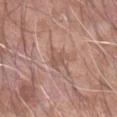Clinical impression: Recorded during total-body skin imaging; not selected for excision or biopsy. Context: The patient is a male about 60 years old. Imaged with white-light lighting. From the right forearm. A 15 mm close-up extracted from a 3D total-body photography capture.